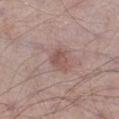Part of a total-body skin-imaging series; this lesion was reviewed on a skin check and was not flagged for biopsy.
A 15 mm close-up tile from a total-body photography series done for melanoma screening.
The lesion is located on the leg.
A male patient aged around 55.
Captured under white-light illumination.
About 2.5 mm across.